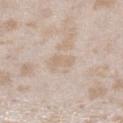Case summary:
* acquisition · 15 mm crop, total-body photography
* anatomic site · the left lower leg
* subject · female, aged 23–27
* lesion diameter · ≈3 mm